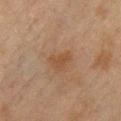workup — total-body-photography surveillance lesion; no biopsy
site — the chest
image source — ~15 mm tile from a whole-body skin photo
subject — female, roughly 40 years of age